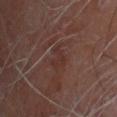Captured during whole-body skin photography for melanoma surveillance; the lesion was not biopsied. A male patient, in their mid-60s. Captured under cross-polarized illumination. The recorded lesion diameter is about 2.5 mm. Located on the head or neck. Automated tile analysis of the lesion measured a mean CIELAB color near L≈31 a*≈19 b*≈22 and a lesion–skin lightness drop of about 4. A 15 mm close-up extracted from a 3D total-body photography capture.